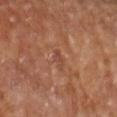workup = imaged on a skin check; not biopsied | image source = 15 mm crop, total-body photography | patient = female, about 70 years old | body site = the left forearm.